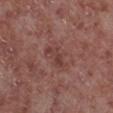• notes · catalogued during a skin exam; not biopsied
• image source · ~15 mm tile from a whole-body skin photo
• site · the leg
• patient · male, in their mid-50s